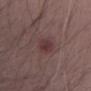Q: Is there a histopathology result?
A: catalogued during a skin exam; not biopsied
Q: Automated lesion metrics?
A: two-axis asymmetry of about 0.15; a border-irregularity index near 2/10, a color-variation rating of about 3/10, and radial color variation of about 1; an automated nevus-likeness rating near 75 out of 100 and a lesion-detection confidence of about 100/100
Q: What lighting was used for the tile?
A: white-light
Q: What is the imaging modality?
A: ~15 mm crop, total-body skin-cancer survey
Q: Who is the patient?
A: male, aged 28–32
Q: How large is the lesion?
A: ~3 mm (longest diameter)